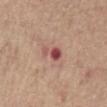This lesion was catalogued during total-body skin photography and was not selected for biopsy. A 15 mm crop from a total-body photograph taken for skin-cancer surveillance. From the abdomen. A male patient, roughly 65 years of age.